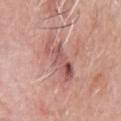biopsy status=total-body-photography surveillance lesion; no biopsy